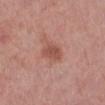The lesion was tiled from a total-body skin photograph and was not biopsied. A close-up tile cropped from a whole-body skin photograph, about 15 mm across. On the left lower leg. A female subject, aged 38 to 42. Imaged with white-light lighting.